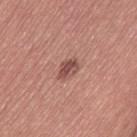diameter: ≈3 mm
image source: ~15 mm crop, total-body skin-cancer survey
patient: female, about 60 years old
illumination: white-light
body site: the left thigh
image-analysis metrics: a lesion area of about 4 mm² and an eccentricity of roughly 0.75; a border-irregularity index near 2/10